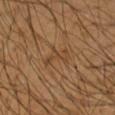biopsy_status: not biopsied; imaged during a skin examination
lesion_size:
  long_diameter_mm_approx: 3.5
lighting: cross-polarized
patient:
  sex: male
  age_approx: 65
automated_metrics:
  color_variation_0_10: 0.0
  peripheral_color_asymmetry: 0.0
  nevus_likeness_0_100: 0
image:
  source: total-body photography crop
  field_of_view_mm: 15
site: right forearm